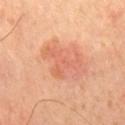Part of a total-body skin-imaging series; this lesion was reviewed on a skin check and was not flagged for biopsy. A 15 mm close-up tile from a total-body photography series done for melanoma screening. A male patient in their mid-60s.